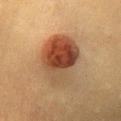Captured during whole-body skin photography for melanoma surveillance; the lesion was not biopsied.
The patient is a female about 55 years old.
On the chest.
This is a cross-polarized tile.
Cropped from a total-body skin-imaging series; the visible field is about 15 mm.
Measured at roughly 6.5 mm in maximum diameter.